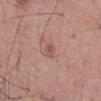follow-up=total-body-photography surveillance lesion; no biopsy | patient=male, about 55 years old | anatomic site=the mid back | acquisition=total-body-photography crop, ~15 mm field of view.